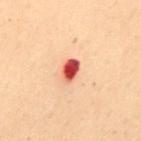  biopsy_status: not biopsied; imaged during a skin examination
  image:
    source: total-body photography crop
    field_of_view_mm: 15
  lesion_size:
    long_diameter_mm_approx: 2.5
  patient:
    sex: female
    age_approx: 50
  lighting: cross-polarized
  automated_metrics:
    vs_skin_darker_L: 21.0
    vs_skin_contrast_norm: 13.5
    color_variation_0_10: 6.0
    peripheral_color_asymmetry: 2.0
    nevus_likeness_0_100: 0
    lesion_detection_confidence_0_100: 100
  site: mid back